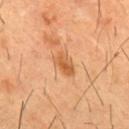Assessment:
The lesion was photographed on a routine skin check and not biopsied; there is no pathology result.
Background:
A roughly 15 mm field-of-view crop from a total-body skin photograph. The lesion-visualizer software estimated an average lesion color of about L≈50 a*≈23 b*≈37 (CIELAB) and a normalized lesion–skin contrast near 7.5. The analysis additionally found a nevus-likeness score of about 50/100 and a detector confidence of about 100 out of 100 that the crop contains a lesion. Imaged with cross-polarized lighting. About 3 mm across. The lesion is located on the chest. The patient is a male in their mid- to late 50s.